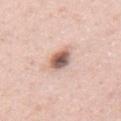The lesion was tiled from a total-body skin photograph and was not biopsied.
A 15 mm close-up tile from a total-body photography series done for melanoma screening.
Approximately 3.5 mm at its widest.
The lesion is on the mid back.
The subject is a male aged approximately 40.
Imaged with white-light lighting.
Automated tile analysis of the lesion measured an eccentricity of roughly 0.7 and two-axis asymmetry of about 0.15. The software also gave border irregularity of about 1.5 on a 0–10 scale and internal color variation of about 9 on a 0–10 scale.